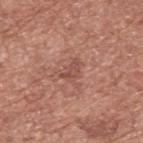workup: catalogued during a skin exam; not biopsied
anatomic site: the upper back
subject: male, aged 73 to 77
acquisition: ~15 mm crop, total-body skin-cancer survey